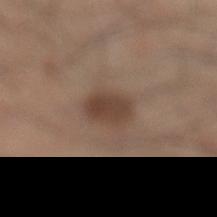This lesion was catalogued during total-body skin photography and was not selected for biopsy. Approximately 3.5 mm at its widest. Located on the left lower leg. A male patient about 35 years old. A region of skin cropped from a whole-body photographic capture, roughly 15 mm wide. Captured under white-light illumination.